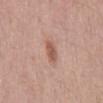Findings:
• workup — imaged on a skin check; not biopsied
• image — ~15 mm crop, total-body skin-cancer survey
• subject — male, approximately 55 years of age
• TBP lesion metrics — a lesion color around L≈56 a*≈21 b*≈28 in CIELAB and a normalized border contrast of about 7; a border-irregularity rating of about 2.5/10, internal color variation of about 3 on a 0–10 scale, and a peripheral color-asymmetry measure near 1; lesion-presence confidence of about 100/100
• location — the mid back